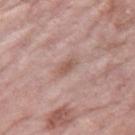biopsy_status: not biopsied; imaged during a skin examination
image:
  source: total-body photography crop
  field_of_view_mm: 15
site: right thigh
lesion_size:
  long_diameter_mm_approx: 2.5
patient:
  sex: female
  age_approx: 70